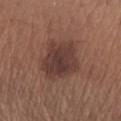Clinical impression: The lesion was photographed on a routine skin check and not biopsied; there is no pathology result.